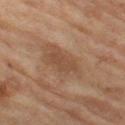The lesion was tiled from a total-body skin photograph and was not biopsied. Longest diameter approximately 4.5 mm. A 15 mm close-up tile from a total-body photography series done for melanoma screening. The lesion is on the left thigh. The patient is a female aged around 70. Automated tile analysis of the lesion measured a footprint of about 6.5 mm², a shape eccentricity near 0.9, and a symmetry-axis asymmetry near 0.35. And it measured a lesion color around L≈45 a*≈19 b*≈29 in CIELAB and a lesion-to-skin contrast of about 6 (normalized; higher = more distinct).